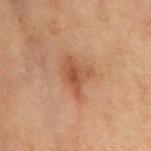Findings:
– follow-up · no biopsy performed (imaged during a skin exam)
– image · ~15 mm crop, total-body skin-cancer survey
– patient · male, approximately 70 years of age
– site · the chest
– lesion diameter · ≈5 mm